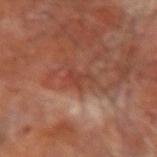  biopsy_status: not biopsied; imaged during a skin examination
  automated_metrics:
    color_variation_0_10: 1.0
    peripheral_color_asymmetry: 0.5
  site: right forearm
  image:
    source: total-body photography crop
    field_of_view_mm: 15
  lighting: cross-polarized
  lesion_size:
    long_diameter_mm_approx: 3.0
  patient:
    sex: male
    age_approx: 65